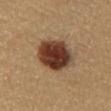notes = imaged on a skin check; not biopsied
subject = female, in their mid- to late 40s
tile lighting = cross-polarized
size = ~5 mm (longest diameter)
acquisition = ~15 mm tile from a whole-body skin photo
location = the front of the torso